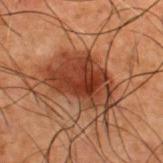The patient is a male about 50 years old. On the back. Cropped from a total-body skin-imaging series; the visible field is about 15 mm. The tile uses cross-polarized illumination.